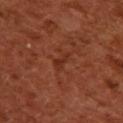Case summary:
• image · ~15 mm tile from a whole-body skin photo
• location · the upper back
• patient · female, approximately 55 years of age
• tile lighting · cross-polarized illumination
• size · ≈2.5 mm